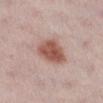notes = catalogued during a skin exam; not biopsied
imaging modality = total-body-photography crop, ~15 mm field of view
patient = female, approximately 30 years of age
tile lighting = white-light illumination
location = the leg